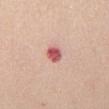workup=total-body-photography surveillance lesion; no biopsy | location=the front of the torso | subject=male, roughly 45 years of age | image=total-body-photography crop, ~15 mm field of view | tile lighting=white-light | lesion diameter=about 2.5 mm.